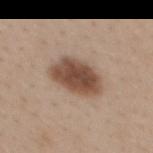Recorded during total-body skin imaging; not selected for excision or biopsy. This image is a 15 mm lesion crop taken from a total-body photograph. Imaged with white-light lighting. Measured at roughly 6 mm in maximum diameter. From the upper back. A female patient aged around 30.